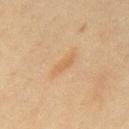Recorded during total-body skin imaging; not selected for excision or biopsy.
A 15 mm close-up tile from a total-body photography series done for melanoma screening.
Approximately 3.5 mm at its widest.
A male subject, aged 43 to 47.
Captured under cross-polarized illumination.
On the mid back.
The total-body-photography lesion software estimated a footprint of about 3 mm², an eccentricity of roughly 0.95, and a shape-asymmetry score of about 0.3 (0 = symmetric). The software also gave a nevus-likeness score of about 10/100.